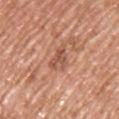Q: Was a biopsy performed?
A: catalogued during a skin exam; not biopsied
Q: Illumination type?
A: white-light illumination
Q: How large is the lesion?
A: ≈3.5 mm
Q: Lesion location?
A: the back
Q: What did automated image analysis measure?
A: an area of roughly 5.5 mm², an outline eccentricity of about 0.8 (0 = round, 1 = elongated), and two-axis asymmetry of about 0.5; a nevus-likeness score of about 0/100 and lesion-presence confidence of about 100/100
Q: What is the imaging modality?
A: ~15 mm crop, total-body skin-cancer survey
Q: What are the patient's age and sex?
A: male, about 70 years old